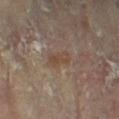Recorded during total-body skin imaging; not selected for excision or biopsy.